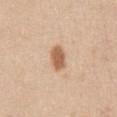Image and clinical context: The patient is a female about 40 years old. Automated tile analysis of the lesion measured a lesion color around L≈60 a*≈21 b*≈35 in CIELAB and a normalized lesion–skin contrast near 9. And it measured a border-irregularity rating of about 1.5/10, a color-variation rating of about 2/10, and radial color variation of about 0.5. The lesion is located on the chest. Approximately 3 mm at its widest. A roughly 15 mm field-of-view crop from a total-body skin photograph.